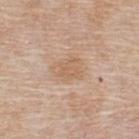Imaged during a routine full-body skin examination; the lesion was not biopsied and no histopathology is available.
This is a white-light tile.
Longest diameter approximately 3 mm.
A close-up tile cropped from a whole-body skin photograph, about 15 mm across.
The subject is a male about 55 years old.
On the upper back.